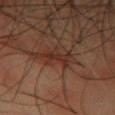The lesion was tiled from a total-body skin photograph and was not biopsied.
The lesion is on the chest.
Longest diameter approximately 3.5 mm.
A male patient roughly 50 years of age.
A 15 mm close-up tile from a total-body photography series done for melanoma screening.